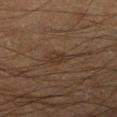Recorded during total-body skin imaging; not selected for excision or biopsy. A male subject in their mid-60s. Cropped from a whole-body photographic skin survey; the tile spans about 15 mm. Located on the right lower leg.